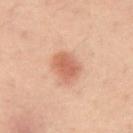follow-up = total-body-photography surveillance lesion; no biopsy
image source = ~15 mm crop, total-body skin-cancer survey
subject = male, roughly 40 years of age
location = the abdomen